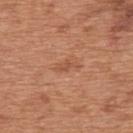{"biopsy_status": "not biopsied; imaged during a skin examination", "site": "upper back", "image": {"source": "total-body photography crop", "field_of_view_mm": 15}, "lesion_size": {"long_diameter_mm_approx": 3.0}, "patient": {"sex": "male", "age_approx": 65}}